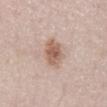Case summary:
• workup · catalogued during a skin exam; not biopsied